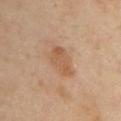{
  "biopsy_status": "not biopsied; imaged during a skin examination",
  "patient": {
    "sex": "female",
    "age_approx": 45
  },
  "automated_metrics": {
    "area_mm2_approx": 8.0,
    "eccentricity": 0.85,
    "shape_asymmetry": 0.2
  },
  "site": "left upper arm",
  "lesion_size": {
    "long_diameter_mm_approx": 4.5
  },
  "image": {
    "source": "total-body photography crop",
    "field_of_view_mm": 15
  },
  "lighting": "cross-polarized"
}